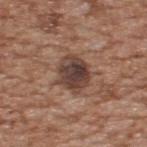notes: total-body-photography surveillance lesion; no biopsy
TBP lesion metrics: a mean CIELAB color near L≈41 a*≈18 b*≈23, a lesion–skin lightness drop of about 13, and a lesion-to-skin contrast of about 10.5 (normalized; higher = more distinct); a nevus-likeness score of about 5/100
diameter: ~4 mm (longest diameter)
tile lighting: white-light illumination
image: 15 mm crop, total-body photography
body site: the upper back
subject: male, aged around 65